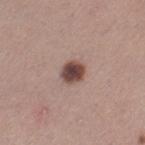workup = total-body-photography surveillance lesion; no biopsy | image source = ~15 mm tile from a whole-body skin photo | body site = the left thigh | subject = female, aged 28 to 32.